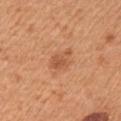notes: catalogued during a skin exam; not biopsied
anatomic site: the chest
automated metrics: a lesion area of about 4.5 mm² and a shape eccentricity near 0.75; an average lesion color of about L≈55 a*≈26 b*≈37 (CIELAB), about 9 CIELAB-L* units darker than the surrounding skin, and a lesion-to-skin contrast of about 6 (normalized; higher = more distinct); a border-irregularity rating of about 3/10, internal color variation of about 2.5 on a 0–10 scale, and peripheral color asymmetry of about 1
lesion diameter: about 3 mm
lighting: white-light
patient: male, aged approximately 55
image: ~15 mm crop, total-body skin-cancer survey